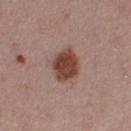Impression:
This lesion was catalogued during total-body skin photography and was not selected for biopsy.
Clinical summary:
An algorithmic analysis of the crop reported a border-irregularity index near 1.5/10, a within-lesion color-variation index near 3/10, and peripheral color asymmetry of about 1. And it measured a classifier nevus-likeness of about 100/100 and lesion-presence confidence of about 100/100. Cropped from a total-body skin-imaging series; the visible field is about 15 mm. This is a white-light tile. Located on the leg. About 4 mm across. A female patient, in their mid-50s.